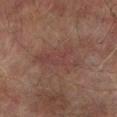Q: Is there a histopathology result?
A: no biopsy performed (imaged during a skin exam)
Q: How was the tile lit?
A: cross-polarized
Q: What is the imaging modality?
A: ~15 mm crop, total-body skin-cancer survey
Q: Automated lesion metrics?
A: a footprint of about 13 mm², an outline eccentricity of about 0.85 (0 = round, 1 = elongated), and a symmetry-axis asymmetry near 0.45; an average lesion color of about L≈33 a*≈17 b*≈19 (CIELAB) and a lesion–skin lightness drop of about 4; a border-irregularity index near 6.5/10, internal color variation of about 2.5 on a 0–10 scale, and radial color variation of about 1
Q: What is the lesion's diameter?
A: ~6 mm (longest diameter)
Q: Who is the patient?
A: male, in their mid- to late 70s
Q: Lesion location?
A: the left lower leg